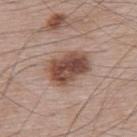This lesion was catalogued during total-body skin photography and was not selected for biopsy.
A lesion tile, about 15 mm wide, cut from a 3D total-body photograph.
The lesion is on the upper back.
The tile uses white-light illumination.
A male patient, aged 63–67.